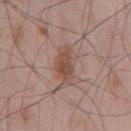  biopsy_status: not biopsied; imaged during a skin examination
  patient:
    sex: male
    age_approx: 55
  site: abdomen
  lighting: white-light
  automated_metrics:
    border_irregularity_0_10: 2.5
    nevus_likeness_0_100: 65
    lesion_detection_confidence_0_100: 100
  image:
    source: total-body photography crop
    field_of_view_mm: 15
  lesion_size:
    long_diameter_mm_approx: 4.5